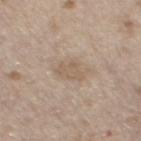Assessment:
This lesion was catalogued during total-body skin photography and was not selected for biopsy.
Acquisition and patient details:
This image is a 15 mm lesion crop taken from a total-body photograph. Captured under white-light illumination. From the left thigh. About 3 mm across. A male subject aged 68–72.